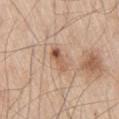workup: no biopsy performed (imaged during a skin exam)
acquisition: ~15 mm tile from a whole-body skin photo
location: the right thigh
illumination: white-light
lesion size: ≈3 mm
image-analysis metrics: a footprint of about 4 mm², an outline eccentricity of about 0.9 (0 = round, 1 = elongated), and two-axis asymmetry of about 0.35; a lesion color around L≈57 a*≈21 b*≈31 in CIELAB, about 11 CIELAB-L* units darker than the surrounding skin, and a lesion-to-skin contrast of about 7.5 (normalized; higher = more distinct); border irregularity of about 3.5 on a 0–10 scale, internal color variation of about 9.5 on a 0–10 scale, and radial color variation of about 3.5; a classifier nevus-likeness of about 55/100 and a lesion-detection confidence of about 100/100
patient: male, aged approximately 60